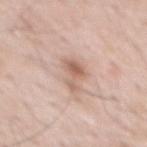<case>
  <image>
    <source>total-body photography crop</source>
    <field_of_view_mm>15</field_of_view_mm>
  </image>
  <lesion_size>
    <long_diameter_mm_approx>4.5</long_diameter_mm_approx>
  </lesion_size>
  <site>mid back</site>
  <patient>
    <sex>male</sex>
    <age_approx>60</age_approx>
  </patient>
</case>